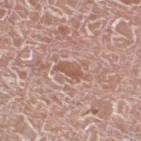biopsy_status: not biopsied; imaged during a skin examination
automated_metrics:
  border_irregularity_0_10: 4.0
  color_variation_0_10: 1.5
  peripheral_color_asymmetry: 0.5
  nevus_likeness_0_100: 0
  lesion_detection_confidence_0_100: 85
site: left lower leg
lighting: white-light
image:
  source: total-body photography crop
  field_of_view_mm: 15
patient:
  sex: male
  age_approx: 75